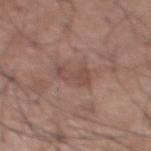Captured during whole-body skin photography for melanoma surveillance; the lesion was not biopsied.
Longest diameter approximately 3 mm.
The lesion is located on the front of the torso.
A 15 mm close-up tile from a total-body photography series done for melanoma screening.
A male subject aged approximately 55.
An algorithmic analysis of the crop reported a footprint of about 4.5 mm² and an outline eccentricity of about 0.8 (0 = round, 1 = elongated). The software also gave a mean CIELAB color near L≈47 a*≈19 b*≈23, about 7 CIELAB-L* units darker than the surrounding skin, and a lesion-to-skin contrast of about 6 (normalized; higher = more distinct). The analysis additionally found an automated nevus-likeness rating near 0 out of 100 and lesion-presence confidence of about 100/100.